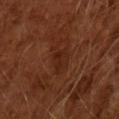notes = catalogued during a skin exam; not biopsied | subject = male, in their mid- to late 60s | automated lesion analysis = a shape eccentricity near 0.9; an automated nevus-likeness rating near 0 out of 100 | image source = ~15 mm crop, total-body skin-cancer survey | lesion size = ~3 mm (longest diameter) | illumination = cross-polarized illumination.